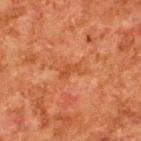follow-up = catalogued during a skin exam; not biopsied | imaging modality = ~15 mm crop, total-body skin-cancer survey | subject = male, roughly 80 years of age | location = the upper back.